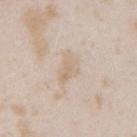Assessment: No biopsy was performed on this lesion — it was imaged during a full skin examination and was not determined to be concerning. Image and clinical context: A female patient aged approximately 25. From the right upper arm. A 15 mm crop from a total-body photograph taken for skin-cancer surveillance.